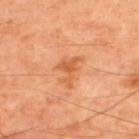Notes:
- workup — catalogued during a skin exam; not biopsied
- image — 15 mm crop, total-body photography
- lesion diameter — ~4 mm (longest diameter)
- body site — the upper back
- patient — male, in their 60s
- tile lighting — cross-polarized